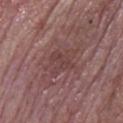This lesion was catalogued during total-body skin photography and was not selected for biopsy.
Cropped from a whole-body photographic skin survey; the tile spans about 15 mm.
On the head or neck.
The subject is a male in their mid-80s or older.
Captured under white-light illumination.
Measured at roughly 4.5 mm in maximum diameter.